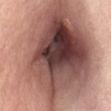Impression:
This lesion was catalogued during total-body skin photography and was not selected for biopsy.
Image and clinical context:
Imaged with white-light lighting. A female subject, in their mid- to late 60s. About 22.5 mm across. Located on the abdomen. A region of skin cropped from a whole-body photographic capture, roughly 15 mm wide.